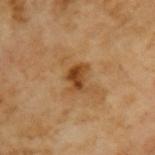– workup · catalogued during a skin exam; not biopsied
– subject · male, in their mid- to late 60s
– size · ≈3 mm
– illumination · cross-polarized illumination
– acquisition · total-body-photography crop, ~15 mm field of view